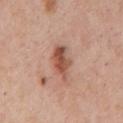Clinical impression: Part of a total-body skin-imaging series; this lesion was reviewed on a skin check and was not flagged for biopsy. Context: Measured at roughly 4.5 mm in maximum diameter. The total-body-photography lesion software estimated a footprint of about 8.5 mm², an eccentricity of roughly 0.8, and a symmetry-axis asymmetry near 0.25. The software also gave a border-irregularity rating of about 3/10, a within-lesion color-variation index near 6/10, and a peripheral color-asymmetry measure near 2. From the mid back. A lesion tile, about 15 mm wide, cut from a 3D total-body photograph. A male patient aged 53 to 57.